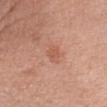Q: Who is the patient?
A: female, aged around 40
Q: Lesion location?
A: the right upper arm
Q: How was this image acquired?
A: ~15 mm crop, total-body skin-cancer survey